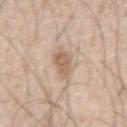Findings:
* notes: imaged on a skin check; not biopsied
* automated metrics: an eccentricity of roughly 0.75 and two-axis asymmetry of about 0.25; a mean CIELAB color near L≈61 a*≈16 b*≈29 and a lesion–skin lightness drop of about 11; a border-irregularity index near 3/10, a within-lesion color-variation index near 2/10, and radial color variation of about 0.5; a nevus-likeness score of about 45/100
* illumination: white-light
* image: total-body-photography crop, ~15 mm field of view
* patient: male, aged approximately 55